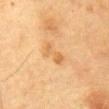workup: catalogued during a skin exam; not biopsied.